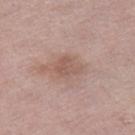Findings:
– notes — catalogued during a skin exam; not biopsied
– image source — ~15 mm tile from a whole-body skin photo
– tile lighting — white-light
– patient — male, approximately 60 years of age
– automated lesion analysis — a lesion area of about 6.5 mm², an eccentricity of roughly 0.65, and two-axis asymmetry of about 0.25; a within-lesion color-variation index near 2.5/10; lesion-presence confidence of about 100/100
– location — the right thigh